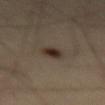Q: Who is the patient?
A: male, aged 63–67
Q: How was this image acquired?
A: ~15 mm tile from a whole-body skin photo
Q: What lighting was used for the tile?
A: cross-polarized
Q: How large is the lesion?
A: ≈2.5 mm
Q: What is the anatomic site?
A: the right thigh